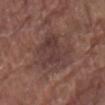Clinical impression: No biopsy was performed on this lesion — it was imaged during a full skin examination and was not determined to be concerning. Context: The recorded lesion diameter is about 5.5 mm. A roughly 15 mm field-of-view crop from a total-body skin photograph. From the lower back. The subject is a male aged 78 to 82. This is a white-light tile.